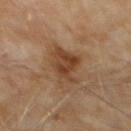Q: Was this lesion biopsied?
A: no biopsy performed (imaged during a skin exam)
Q: What is the lesion's diameter?
A: ≈5.5 mm
Q: Patient demographics?
A: male, roughly 60 years of age
Q: Illumination type?
A: cross-polarized
Q: Automated lesion metrics?
A: a shape eccentricity near 0.85 and a symmetry-axis asymmetry near 0.35; border irregularity of about 4 on a 0–10 scale and a peripheral color-asymmetry measure near 1.5; an automated nevus-likeness rating near 5 out of 100 and lesion-presence confidence of about 100/100
Q: How was this image acquired?
A: ~15 mm tile from a whole-body skin photo
Q: Lesion location?
A: the abdomen